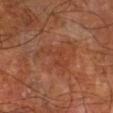Clinical summary:
Automated image analysis of the tile measured a lesion area of about 6 mm², an outline eccentricity of about 0.85 (0 = round, 1 = elongated), and two-axis asymmetry of about 0.55. And it measured a mean CIELAB color near L≈40 a*≈26 b*≈32, a lesion–skin lightness drop of about 5, and a lesion-to-skin contrast of about 4.5 (normalized; higher = more distinct). It also reported an automated nevus-likeness rating near 0 out of 100 and lesion-presence confidence of about 95/100. Imaged with cross-polarized lighting. The lesion's longest dimension is about 4.5 mm. Located on the leg. A male patient, aged approximately 60. A 15 mm crop from a total-body photograph taken for skin-cancer surveillance.